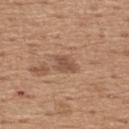Imaged during a routine full-body skin examination; the lesion was not biopsied and no histopathology is available.
A region of skin cropped from a whole-body photographic capture, roughly 15 mm wide.
On the upper back.
A male subject aged around 65.
Longest diameter approximately 3 mm.
Imaged with white-light lighting.